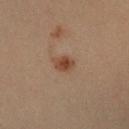Q: Is there a histopathology result?
A: catalogued during a skin exam; not biopsied
Q: Lesion size?
A: about 3 mm
Q: What is the imaging modality?
A: total-body-photography crop, ~15 mm field of view
Q: Illumination type?
A: cross-polarized
Q: What are the patient's age and sex?
A: male, approximately 35 years of age
Q: Lesion location?
A: the arm